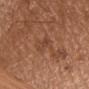A male subject, aged approximately 65. A 15 mm close-up tile from a total-body photography series done for melanoma screening. Located on the chest.